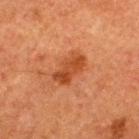| key | value |
|---|---|
| workup | catalogued during a skin exam; not biopsied |
| body site | the upper back |
| tile lighting | cross-polarized illumination |
| image | 15 mm crop, total-body photography |
| size | ~4.5 mm (longest diameter) |
| patient | male, approximately 50 years of age |